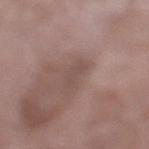- notes — catalogued during a skin exam; not biopsied
- automated lesion analysis — an area of roughly 3 mm², an outline eccentricity of about 0.85 (0 = round, 1 = elongated), and a shape-asymmetry score of about 0.45 (0 = symmetric); a border-irregularity index near 5/10, a within-lesion color-variation index near 0.5/10, and radial color variation of about 0; a classifier nevus-likeness of about 0/100 and a detector confidence of about 85 out of 100 that the crop contains a lesion
- image — ~15 mm tile from a whole-body skin photo
- lighting — white-light
- site — the left lower leg
- subject — female, aged approximately 70
- lesion diameter — about 2.5 mm